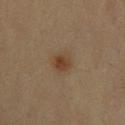notes: catalogued during a skin exam; not biopsied
body site: the upper back
image-analysis metrics: an average lesion color of about L≈36 a*≈15 b*≈27 (CIELAB), roughly 7 lightness units darker than nearby skin, and a lesion-to-skin contrast of about 7.5 (normalized; higher = more distinct); internal color variation of about 3 on a 0–10 scale and peripheral color asymmetry of about 1; a lesion-detection confidence of about 100/100
illumination: cross-polarized
patient: male, aged approximately 35
lesion size: about 2.5 mm
acquisition: total-body-photography crop, ~15 mm field of view